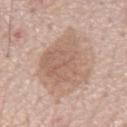– workup · total-body-photography surveillance lesion; no biopsy
– lesion diameter · ≈7 mm
– subject · male, in their 70s
– anatomic site · the chest
– image · ~15 mm crop, total-body skin-cancer survey
– lighting · white-light